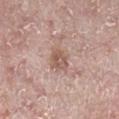Recorded during total-body skin imaging; not selected for excision or biopsy. The patient is a male in their mid- to late 60s. A 15 mm crop from a total-body photograph taken for skin-cancer surveillance. Located on the right lower leg.